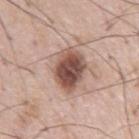  biopsy_status: not biopsied; imaged during a skin examination
  lighting: white-light
  image:
    source: total-body photography crop
    field_of_view_mm: 15
  automated_metrics:
    area_mm2_approx: 14.0
    eccentricity: 0.65
    shape_asymmetry: 0.1
    vs_skin_darker_L: 17.0
    vs_skin_contrast_norm: 11.5
    peripheral_color_asymmetry: 2.0
    nevus_likeness_0_100: 85
  patient:
    sex: male
    age_approx: 55